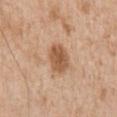Q: Was a biopsy performed?
A: imaged on a skin check; not biopsied
Q: What is the lesion's diameter?
A: about 4 mm
Q: What kind of image is this?
A: ~15 mm crop, total-body skin-cancer survey
Q: Illumination type?
A: white-light illumination
Q: What did automated image analysis measure?
A: a lesion area of about 8.5 mm², an eccentricity of roughly 0.75, and a symmetry-axis asymmetry near 0.15; an average lesion color of about L≈56 a*≈21 b*≈34 (CIELAB)
Q: What is the anatomic site?
A: the chest
Q: What are the patient's age and sex?
A: male, aged approximately 65